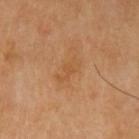Case summary:
• notes · catalogued during a skin exam; not biopsied
• image source · total-body-photography crop, ~15 mm field of view
• location · the left upper arm
• patient · male, aged around 70